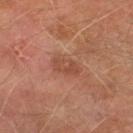Context: Automated image analysis of the tile measured a footprint of about 5 mm² and an outline eccentricity of about 0.9 (0 = round, 1 = elongated). The software also gave a mean CIELAB color near L≈38 a*≈21 b*≈26, a lesion–skin lightness drop of about 6, and a normalized lesion–skin contrast near 5.5. The analysis additionally found border irregularity of about 2.5 on a 0–10 scale, a within-lesion color-variation index near 2.5/10, and radial color variation of about 1. Cropped from a total-body skin-imaging series; the visible field is about 15 mm. A male subject about 75 years old. Located on the left thigh.